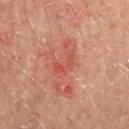This lesion was catalogued during total-body skin photography and was not selected for biopsy. The lesion is on the mid back. The lesion-visualizer software estimated a lesion–skin lightness drop of about 6 and a lesion-to-skin contrast of about 5 (normalized; higher = more distinct). A male patient, aged 63 to 67. Imaged with cross-polarized lighting. Longest diameter approximately 3.5 mm. A region of skin cropped from a whole-body photographic capture, roughly 15 mm wide.